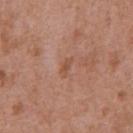No biopsy was performed on this lesion — it was imaged during a full skin examination and was not determined to be concerning.
The lesion's longest dimension is about 2.5 mm.
The tile uses white-light illumination.
A male subject roughly 75 years of age.
A 15 mm close-up extracted from a 3D total-body photography capture.
On the chest.